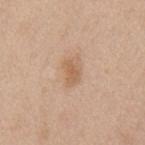- notes: total-body-photography surveillance lesion; no biopsy